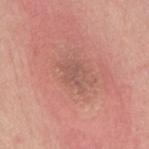{"biopsy_status": "not biopsied; imaged during a skin examination", "lighting": "white-light", "site": "mid back", "automated_metrics": {"cielab_L": 54, "cielab_a": 23, "cielab_b": 26, "vs_skin_darker_L": 6.0, "nevus_likeness_0_100": 0, "lesion_detection_confidence_0_100": 95}, "patient": {"sex": "female", "age_approx": 55}, "lesion_size": {"long_diameter_mm_approx": 3.5}, "image": {"source": "total-body photography crop", "field_of_view_mm": 15}}